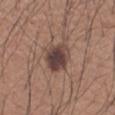The total-body-photography lesion software estimated a footprint of about 10 mm². The software also gave border irregularity of about 1.5 on a 0–10 scale, a within-lesion color-variation index near 6/10, and peripheral color asymmetry of about 1.5.
A 15 mm close-up tile from a total-body photography series done for melanoma screening.
Imaged with white-light lighting.
About 4 mm across.
From the arm.
The patient is a male about 60 years old.